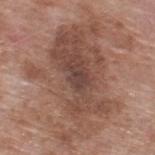Q: Was this lesion biopsied?
A: no biopsy performed (imaged during a skin exam)
Q: Lesion location?
A: the upper back
Q: Who is the patient?
A: male, about 60 years old
Q: How was this image acquired?
A: ~15 mm tile from a whole-body skin photo